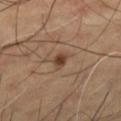* workup · no biopsy performed (imaged during a skin exam)
* patient · male, aged approximately 65
* body site · the leg
* image · total-body-photography crop, ~15 mm field of view
* TBP lesion metrics · a border-irregularity rating of about 3/10, a color-variation rating of about 1.5/10, and peripheral color asymmetry of about 0.5; an automated nevus-likeness rating near 95 out of 100